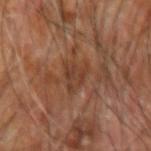Clinical summary: A male subject in their mid- to late 60s. A lesion tile, about 15 mm wide, cut from a 3D total-body photograph. Imaged with cross-polarized lighting. The lesion is on the right forearm. The lesion-visualizer software estimated roughly 7 lightness units darker than nearby skin and a normalized border contrast of about 6. The analysis additionally found an automated nevus-likeness rating near 0 out of 100 and lesion-presence confidence of about 75/100. The recorded lesion diameter is about 3 mm.